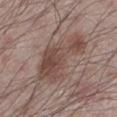follow-up = no biopsy performed (imaged during a skin exam) | anatomic site = the left lower leg | subject = male, aged around 60 | illumination = white-light | automated metrics = a border-irregularity rating of about 6.5/10, a color-variation rating of about 4.5/10, and peripheral color asymmetry of about 1.5; a nevus-likeness score of about 5/100 and a lesion-detection confidence of about 100/100 | lesion size = ~8 mm (longest diameter) | acquisition = ~15 mm crop, total-body skin-cancer survey.